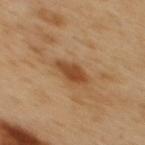Acquisition and patient details: The tile uses cross-polarized illumination. A male patient, in their 50s. The lesion is on the mid back. A roughly 15 mm field-of-view crop from a total-body skin photograph. The recorded lesion diameter is about 3.5 mm.